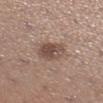biopsy_status: not biopsied; imaged during a skin examination
lesion_size:
  long_diameter_mm_approx: 4.0
image:
  source: total-body photography crop
  field_of_view_mm: 15
patient:
  sex: female
  age_approx: 30
lighting: white-light
site: right lower leg
automated_metrics:
  eccentricity: 0.65
  cielab_L: 49
  cielab_a: 17
  cielab_b: 23
  vs_skin_darker_L: 11.0
  vs_skin_contrast_norm: 8.0
  border_irregularity_0_10: 2.0
  color_variation_0_10: 5.0
  peripheral_color_asymmetry: 1.5
  nevus_likeness_0_100: 55